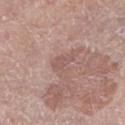This lesion was catalogued during total-body skin photography and was not selected for biopsy. The total-body-photography lesion software estimated border irregularity of about 4 on a 0–10 scale, a color-variation rating of about 0.5/10, and a peripheral color-asymmetry measure near 0. A 15 mm close-up extracted from a 3D total-body photography capture. The patient is a male aged 53–57. On the left lower leg.